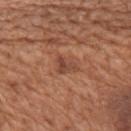Assessment: Recorded during total-body skin imaging; not selected for excision or biopsy. Image and clinical context: An algorithmic analysis of the crop reported an eccentricity of roughly 0.75 and a shape-asymmetry score of about 0.5 (0 = symmetric). And it measured an average lesion color of about L≈45 a*≈23 b*≈30 (CIELAB), a lesion–skin lightness drop of about 9, and a normalized lesion–skin contrast near 6.5. The software also gave a classifier nevus-likeness of about 5/100. About 3 mm across. The patient is a male aged 63 to 67. On the mid back. Imaged with white-light lighting. A 15 mm close-up tile from a total-body photography series done for melanoma screening.